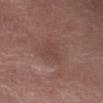  biopsy_status: not biopsied; imaged during a skin examination
  site: abdomen
  automated_metrics:
    cielab_L: 43
    cielab_a: 19
    cielab_b: 22
    vs_skin_darker_L: 5.0
    vs_skin_contrast_norm: 4.5
  patient:
    sex: female
    age_approx: 70
  image:
    source: total-body photography crop
    field_of_view_mm: 15
  lesion_size:
    long_diameter_mm_approx: 3.0